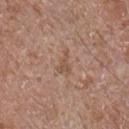Assessment: Imaged during a routine full-body skin examination; the lesion was not biopsied and no histopathology is available. Clinical summary: From the left forearm. A close-up tile cropped from a whole-body skin photograph, about 15 mm across. The total-body-photography lesion software estimated a lesion area of about 3.5 mm², an outline eccentricity of about 0.75 (0 = round, 1 = elongated), and two-axis asymmetry of about 0.55. The software also gave a border-irregularity index near 6/10 and a within-lesion color-variation index near 1/10. This is a white-light tile. The patient is a male aged 58 to 62.